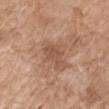Captured during whole-body skin photography for melanoma surveillance; the lesion was not biopsied. The lesion is on the right upper arm. Longest diameter approximately 3.5 mm. A female subject aged approximately 75. A lesion tile, about 15 mm wide, cut from a 3D total-body photograph.